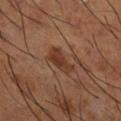No biopsy was performed on this lesion — it was imaged during a full skin examination and was not determined to be concerning. A close-up tile cropped from a whole-body skin photograph, about 15 mm across. From the right lower leg. The subject is a male about 65 years old.